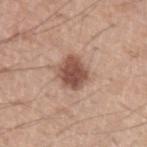This lesion was catalogued during total-body skin photography and was not selected for biopsy. Cropped from a total-body skin-imaging series; the visible field is about 15 mm. A male subject about 55 years old.